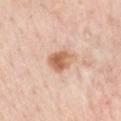{
  "biopsy_status": "not biopsied; imaged during a skin examination"
}